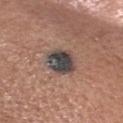{"biopsy_status": "not biopsied; imaged during a skin examination", "patient": {"sex": "female", "age_approx": 55}, "lesion_size": {"long_diameter_mm_approx": 4.0}, "site": "head or neck", "lighting": "white-light", "image": {"source": "total-body photography crop", "field_of_view_mm": 15}}